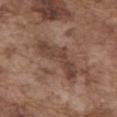Case summary:
* follow-up — imaged on a skin check; not biopsied
* patient — male, aged around 75
* body site — the front of the torso
* image source — 15 mm crop, total-body photography
* size — about 7 mm